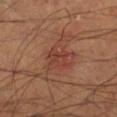<tbp_lesion>
<biopsy_status>not biopsied; imaged during a skin examination</biopsy_status>
<automated_metrics>
  <area_mm2_approx>6.5</area_mm2_approx>
  <eccentricity>0.65</eccentricity>
  <shape_asymmetry>0.4</shape_asymmetry>
  <vs_skin_contrast_norm>5.5</vs_skin_contrast_norm>
  <nevus_likeness_0_100>5</nevus_likeness_0_100>
  <lesion_detection_confidence_0_100>100</lesion_detection_confidence_0_100>
</automated_metrics>
<site>left lower leg</site>
<patient>
  <sex>male</sex>
  <age_approx>65</age_approx>
</patient>
<lesion_size>
  <long_diameter_mm_approx>4.0</long_diameter_mm_approx>
</lesion_size>
<lighting>cross-polarized</lighting>
<image>
  <source>total-body photography crop</source>
  <field_of_view_mm>15</field_of_view_mm>
</image>
</tbp_lesion>